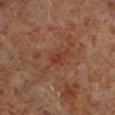biopsy status: imaged on a skin check; not biopsied
site: the left lower leg
size: ≈2.5 mm
automated lesion analysis: a footprint of about 2.5 mm², an eccentricity of roughly 0.85, and a symmetry-axis asymmetry near 0.35; border irregularity of about 3.5 on a 0–10 scale, internal color variation of about 0.5 on a 0–10 scale, and radial color variation of about 0
acquisition: ~15 mm tile from a whole-body skin photo
patient: male, aged 58–62
lighting: cross-polarized illumination